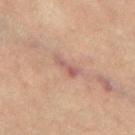Impression: Captured during whole-body skin photography for melanoma surveillance; the lesion was not biopsied. Image and clinical context: The lesion is located on the left thigh. The subject is a female in their mid- to late 60s. Cropped from a total-body skin-imaging series; the visible field is about 15 mm.